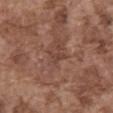Q: Is there a histopathology result?
A: total-body-photography surveillance lesion; no biopsy
Q: Illumination type?
A: white-light illumination
Q: Lesion location?
A: the abdomen
Q: What did automated image analysis measure?
A: a color-variation rating of about 0/10 and peripheral color asymmetry of about 0; an automated nevus-likeness rating near 0 out of 100 and a lesion-detection confidence of about 85/100
Q: What is the imaging modality?
A: total-body-photography crop, ~15 mm field of view
Q: Who is the patient?
A: male, in their mid- to late 70s